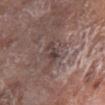Part of a total-body skin-imaging series; this lesion was reviewed on a skin check and was not flagged for biopsy. A 15 mm close-up extracted from a 3D total-body photography capture. Longest diameter approximately 3 mm. Automated tile analysis of the lesion measured an average lesion color of about L≈40 a*≈15 b*≈18 (CIELAB), roughly 8 lightness units darker than nearby skin, and a lesion-to-skin contrast of about 7 (normalized; higher = more distinct). The software also gave a border-irregularity index near 4.5/10 and internal color variation of about 2.5 on a 0–10 scale. The subject is a female in their mid-70s. The lesion is on the chest.